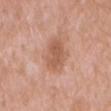Q: Was a biopsy performed?
A: imaged on a skin check; not biopsied
Q: What are the patient's age and sex?
A: male, aged around 60
Q: What kind of image is this?
A: total-body-photography crop, ~15 mm field of view
Q: What lighting was used for the tile?
A: white-light illumination
Q: How large is the lesion?
A: ≈4.5 mm
Q: What is the anatomic site?
A: the abdomen